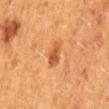Clinical impression: This lesion was catalogued during total-body skin photography and was not selected for biopsy. Background: A 15 mm crop from a total-body photograph taken for skin-cancer surveillance. Automated image analysis of the tile measured a lesion color around L≈46 a*≈25 b*≈36 in CIELAB and about 9 CIELAB-L* units darker than the surrounding skin. The analysis additionally found an automated nevus-likeness rating near 85 out of 100 and lesion-presence confidence of about 100/100. On the lower back. Imaged with cross-polarized lighting. A female subject aged approximately 50.